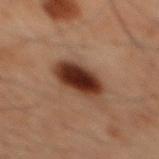Assessment:
Recorded during total-body skin imaging; not selected for excision or biopsy.
Image and clinical context:
A 15 mm close-up tile from a total-body photography series done for melanoma screening. A male subject about 60 years old. About 5 mm across. The tile uses cross-polarized illumination. Located on the mid back.